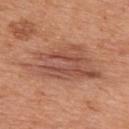Impression: The lesion was tiled from a total-body skin photograph and was not biopsied. Clinical summary: A 15 mm close-up extracted from a 3D total-body photography capture. The subject is a male aged 68 to 72. Approximately 9.5 mm at its widest. This is a white-light tile. Automated tile analysis of the lesion measured a footprint of about 28 mm² and a symmetry-axis asymmetry near 0.35. The analysis additionally found an automated nevus-likeness rating near 0 out of 100. From the upper back.